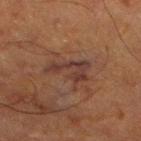biopsy status=imaged on a skin check; not biopsied
diameter=≈5 mm
tile lighting=cross-polarized
body site=the right lower leg
subject=male, aged 63–67
acquisition=15 mm crop, total-body photography